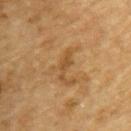| key | value |
|---|---|
| workup | catalogued during a skin exam; not biopsied |